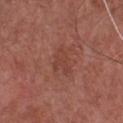Q: Was a biopsy performed?
A: imaged on a skin check; not biopsied
Q: What lighting was used for the tile?
A: white-light illumination
Q: What are the patient's age and sex?
A: male, about 75 years old
Q: What is the anatomic site?
A: the chest
Q: What kind of image is this?
A: ~15 mm crop, total-body skin-cancer survey
Q: What is the lesion's diameter?
A: about 3.5 mm
Q: Automated lesion metrics?
A: a lesion–skin lightness drop of about 6 and a normalized border contrast of about 4.5; internal color variation of about 1 on a 0–10 scale and a peripheral color-asymmetry measure near 0.5